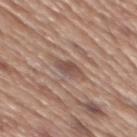Clinical summary: The lesion is located on the mid back. The patient is a male aged 63 to 67. A roughly 15 mm field-of-view crop from a total-body skin photograph.